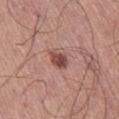Part of a total-body skin-imaging series; this lesion was reviewed on a skin check and was not flagged for biopsy. This image is a 15 mm lesion crop taken from a total-body photograph. The subject is a male aged approximately 65. The lesion is located on the right thigh.